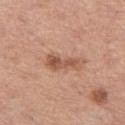Clinical impression: This lesion was catalogued during total-body skin photography and was not selected for biopsy. Context: Imaged with white-light lighting. About 4 mm across. Cropped from a whole-body photographic skin survey; the tile spans about 15 mm. On the right thigh. The patient is a female about 60 years old.